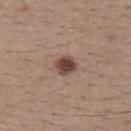Q: Is there a histopathology result?
A: imaged on a skin check; not biopsied
Q: Patient demographics?
A: male, aged approximately 40
Q: Illumination type?
A: white-light
Q: What is the anatomic site?
A: the upper back
Q: How was this image acquired?
A: ~15 mm crop, total-body skin-cancer survey